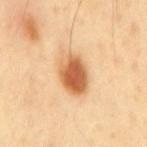Clinical impression: Captured during whole-body skin photography for melanoma surveillance; the lesion was not biopsied. Clinical summary: The subject is a male in their 40s. Cropped from a whole-body photographic skin survey; the tile spans about 15 mm. Located on the mid back. Longest diameter approximately 5 mm. An algorithmic analysis of the crop reported a lesion area of about 13 mm², an eccentricity of roughly 0.8, and a symmetry-axis asymmetry near 0.1. And it measured a mean CIELAB color near L≈64 a*≈24 b*≈41, roughly 16 lightness units darker than nearby skin, and a normalized border contrast of about 9.5.